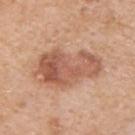Case summary:
• follow-up: total-body-photography surveillance lesion; no biopsy
• subject: male, aged 58 to 62
• image: ~15 mm tile from a whole-body skin photo
• lesion size: about 7.5 mm
• location: the upper back